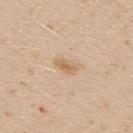Assessment: The lesion was photographed on a routine skin check and not biopsied; there is no pathology result. Context: The patient is a male aged approximately 30. Captured under white-light illumination. Measured at roughly 3 mm in maximum diameter. This image is a 15 mm lesion crop taken from a total-body photograph. Located on the upper back. Automated image analysis of the tile measured an outline eccentricity of about 0.85 (0 = round, 1 = elongated) and a shape-asymmetry score of about 0.2 (0 = symmetric). And it measured a border-irregularity rating of about 2/10, internal color variation of about 2.5 on a 0–10 scale, and peripheral color asymmetry of about 0.5.